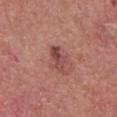workup: catalogued during a skin exam; not biopsied | subject: male, aged 58–62 | illumination: white-light | body site: the head or neck | imaging modality: total-body-photography crop, ~15 mm field of view | size: about 3 mm.